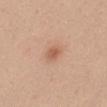Impression:
Captured during whole-body skin photography for melanoma surveillance; the lesion was not biopsied.
Background:
The lesion is located on the mid back. Approximately 2.5 mm at its widest. A female patient in their mid-40s. The lesion-visualizer software estimated a shape eccentricity near 0.65 and a symmetry-axis asymmetry near 0.2. It also reported an average lesion color of about L≈59 a*≈22 b*≈32 (CIELAB), about 9 CIELAB-L* units darker than the surrounding skin, and a normalized lesion–skin contrast near 6. A roughly 15 mm field-of-view crop from a total-body skin photograph. The tile uses white-light illumination.